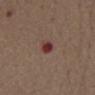Case summary:
– workup · catalogued during a skin exam; not biopsied
– site · the abdomen
– tile lighting · white-light illumination
– patient · male, in their mid- to late 70s
– acquisition · total-body-photography crop, ~15 mm field of view
– lesion size · ~2.5 mm (longest diameter)